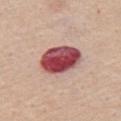Assessment:
Captured during whole-body skin photography for melanoma surveillance; the lesion was not biopsied.
Acquisition and patient details:
A male patient, roughly 65 years of age. A 15 mm close-up extracted from a 3D total-body photography capture. On the abdomen. Longest diameter approximately 5 mm. The lesion-visualizer software estimated a lesion–skin lightness drop of about 22 and a normalized lesion–skin contrast near 14.5. The analysis additionally found a border-irregularity index near 1.5/10 and a peripheral color-asymmetry measure near 3. And it measured a classifier nevus-likeness of about 0/100 and a detector confidence of about 100 out of 100 that the crop contains a lesion. Captured under white-light illumination.